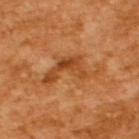Q: Was this lesion biopsied?
A: imaged on a skin check; not biopsied
Q: What is the imaging modality?
A: 15 mm crop, total-body photography
Q: What are the patient's age and sex?
A: male, in their mid- to late 60s
Q: Lesion size?
A: about 6.5 mm
Q: How was the tile lit?
A: cross-polarized illumination